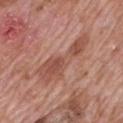| key | value |
|---|---|
| biopsy status | imaged on a skin check; not biopsied |
| patient | male, aged around 70 |
| lesion size | ≈7.5 mm |
| imaging modality | ~15 mm tile from a whole-body skin photo |
| site | the mid back |
| image-analysis metrics | an area of roughly 15 mm², an eccentricity of roughly 0.95, and two-axis asymmetry of about 0.55; about 9 CIELAB-L* units darker than the surrounding skin and a lesion-to-skin contrast of about 6.5 (normalized; higher = more distinct); a border-irregularity rating of about 7/10 and radial color variation of about 1; a classifier nevus-likeness of about 0/100 and a detector confidence of about 100 out of 100 that the crop contains a lesion |
| tile lighting | white-light illumination |